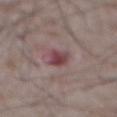{"biopsy_status": "not biopsied; imaged during a skin examination", "lesion_size": {"long_diameter_mm_approx": 3.0}, "automated_metrics": {"cielab_L": 43, "cielab_a": 23, "cielab_b": 16, "vs_skin_darker_L": 11.0, "vs_skin_contrast_norm": 8.5, "border_irregularity_0_10": 2.5, "peripheral_color_asymmetry": 1.5, "lesion_detection_confidence_0_100": 100}, "image": {"source": "total-body photography crop", "field_of_view_mm": 15}, "lighting": "white-light", "patient": {"sex": "male", "age_approx": 75}, "site": "abdomen"}